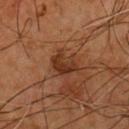No biopsy was performed on this lesion — it was imaged during a full skin examination and was not determined to be concerning.